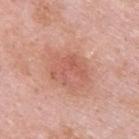Clinical impression:
The lesion was tiled from a total-body skin photograph and was not biopsied.
Acquisition and patient details:
Automated tile analysis of the lesion measured a lesion area of about 7.5 mm² and an eccentricity of roughly 0.65. It also reported an average lesion color of about L≈58 a*≈27 b*≈29 (CIELAB) and about 8 CIELAB-L* units darker than the surrounding skin. It also reported a lesion-detection confidence of about 100/100. From the upper back. Measured at roughly 4 mm in maximum diameter. A lesion tile, about 15 mm wide, cut from a 3D total-body photograph. A male subject about 25 years old.